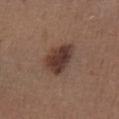Recorded during total-body skin imaging; not selected for excision or biopsy. Captured under white-light illumination. The recorded lesion diameter is about 4.5 mm. A 15 mm crop from a total-body photograph taken for skin-cancer surveillance. A male subject aged 43 to 47. Located on the left lower leg.